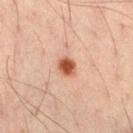{"biopsy_status": "not biopsied; imaged during a skin examination", "site": "right thigh", "patient": {"sex": "male", "age_approx": 35}, "image": {"source": "total-body photography crop", "field_of_view_mm": 15}, "lighting": "cross-polarized", "lesion_size": {"long_diameter_mm_approx": 2.5}}